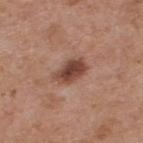notes = catalogued during a skin exam; not biopsied | lighting = white-light | subject = male, in their mid-50s | imaging modality = total-body-photography crop, ~15 mm field of view | location = the upper back.